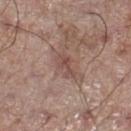Q: Was a biopsy performed?
A: imaged on a skin check; not biopsied
Q: Illumination type?
A: white-light illumination
Q: What is the anatomic site?
A: the right lower leg
Q: Lesion size?
A: about 3 mm
Q: Who is the patient?
A: male, in their 70s
Q: What is the imaging modality?
A: 15 mm crop, total-body photography